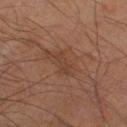notes: imaged on a skin check; not biopsied
anatomic site: the right lower leg
patient: male, roughly 65 years of age
lesion diameter: ~3 mm (longest diameter)
automated metrics: an area of roughly 3 mm² and a shape eccentricity near 0.9; a nevus-likeness score of about 0/100
image: ~15 mm tile from a whole-body skin photo